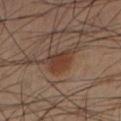<case>
<biopsy_status>not biopsied; imaged during a skin examination</biopsy_status>
<patient>
  <sex>male</sex>
  <age_approx>40</age_approx>
</patient>
<automated_metrics>
  <border_irregularity_0_10>4.5</border_irregularity_0_10>
  <color_variation_0_10>3.5</color_variation_0_10>
  <peripheral_color_asymmetry>1.0</peripheral_color_asymmetry>
</automated_metrics>
<lighting>cross-polarized</lighting>
<image>
  <source>total-body photography crop</source>
  <field_of_view_mm>15</field_of_view_mm>
</image>
<site>left lower leg</site>
<lesion_size>
  <long_diameter_mm_approx>5.0</long_diameter_mm_approx>
</lesion_size>
</case>